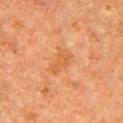The lesion is located on the right upper arm. The subject is a male aged 58 to 62. A region of skin cropped from a whole-body photographic capture, roughly 15 mm wide. The tile uses cross-polarized illumination. The recorded lesion diameter is about 4 mm.